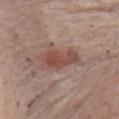Clinical impression: This lesion was catalogued during total-body skin photography and was not selected for biopsy. Image and clinical context: A female subject aged 48–52. Located on the chest. A lesion tile, about 15 mm wide, cut from a 3D total-body photograph.